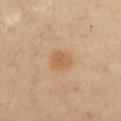Case summary:
* biopsy status — total-body-photography surveillance lesion; no biopsy
* lesion diameter — about 2.5 mm
* location — the chest
* imaging modality — ~15 mm crop, total-body skin-cancer survey
* image-analysis metrics — a nevus-likeness score of about 40/100
* patient — female, in their 40s
* illumination — cross-polarized illumination